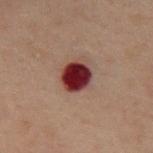Recorded during total-body skin imaging; not selected for excision or biopsy. The lesion-visualizer software estimated a lesion area of about 8 mm², an eccentricity of roughly 0.55, and a shape-asymmetry score of about 0.1 (0 = symmetric). It also reported a mean CIELAB color near L≈23 a*≈25 b*≈17, roughly 19 lightness units darker than nearby skin, and a normalized lesion–skin contrast near 18. The software also gave a border-irregularity index near 1/10, internal color variation of about 4 on a 0–10 scale, and a peripheral color-asymmetry measure near 1.5. The analysis additionally found a classifier nevus-likeness of about 0/100 and lesion-presence confidence of about 100/100. A lesion tile, about 15 mm wide, cut from a 3D total-body photograph. The patient is a male aged around 60. From the back. The tile uses cross-polarized illumination.